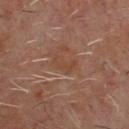Notes:
– follow-up — imaged on a skin check; not biopsied
– acquisition — ~15 mm crop, total-body skin-cancer survey
– patient — male, about 60 years old
– diameter — about 3 mm
– image-analysis metrics — a lesion area of about 3.5 mm², a shape eccentricity near 0.85, and two-axis asymmetry of about 0.5; an average lesion color of about L≈40 a*≈19 b*≈28 (CIELAB) and a lesion–skin lightness drop of about 5; a nevus-likeness score of about 0/100
– site — the chest
– tile lighting — cross-polarized